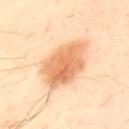Q: Was this lesion biopsied?
A: catalogued during a skin exam; not biopsied
Q: What kind of image is this?
A: ~15 mm tile from a whole-body skin photo
Q: How was the tile lit?
A: cross-polarized
Q: Patient demographics?
A: male, aged approximately 50
Q: What is the anatomic site?
A: the upper back